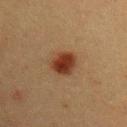Imaged during a routine full-body skin examination; the lesion was not biopsied and no histopathology is available. On the left upper arm. Automated image analysis of the tile measured roughly 12 lightness units darker than nearby skin. The analysis additionally found a border-irregularity index near 1.5/10, internal color variation of about 4.5 on a 0–10 scale, and peripheral color asymmetry of about 1.5. The analysis additionally found a classifier nevus-likeness of about 100/100 and lesion-presence confidence of about 100/100. A male subject, approximately 40 years of age. The lesion's longest dimension is about 3.5 mm. A 15 mm crop from a total-body photograph taken for skin-cancer surveillance.